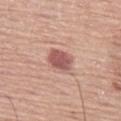The lesion was photographed on a routine skin check and not biopsied; there is no pathology result. The lesion is on the left thigh. A roughly 15 mm field-of-view crop from a total-body skin photograph. A male patient, aged approximately 75.